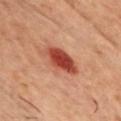notes = imaged on a skin check; not biopsied
subject = male, approximately 50 years of age
automated metrics = an average lesion color of about L≈38 a*≈27 b*≈28 (CIELAB); a border-irregularity index near 2/10 and internal color variation of about 4 on a 0–10 scale; a detector confidence of about 100 out of 100 that the crop contains a lesion
location = the chest
tile lighting = cross-polarized
lesion size = ≈4 mm
acquisition = ~15 mm tile from a whole-body skin photo